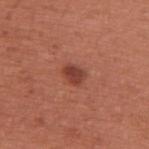Case summary:
– biopsy status — total-body-photography surveillance lesion; no biopsy
– site — the upper back
– patient — female, aged approximately 65
– lesion diameter — ~2.5 mm (longest diameter)
– imaging modality — ~15 mm tile from a whole-body skin photo